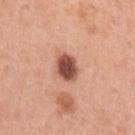Imaged during a routine full-body skin examination; the lesion was not biopsied and no histopathology is available.
The patient is a female roughly 40 years of age.
Located on the left upper arm.
This image is a 15 mm lesion crop taken from a total-body photograph.